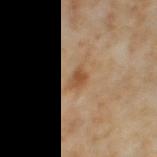{
  "biopsy_status": "not biopsied; imaged during a skin examination",
  "lesion_size": {
    "long_diameter_mm_approx": 2.5
  },
  "image": {
    "source": "total-body photography crop",
    "field_of_view_mm": 15
  },
  "site": "leg",
  "patient": {
    "sex": "female",
    "age_approx": 55
  }
}